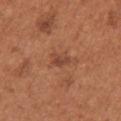Impression: This lesion was catalogued during total-body skin photography and was not selected for biopsy. Background: On the right upper arm. The recorded lesion diameter is about 2.5 mm. Automated image analysis of the tile measured a shape eccentricity near 0.8. The software also gave internal color variation of about 1.5 on a 0–10 scale and a peripheral color-asymmetry measure near 0.5. The software also gave an automated nevus-likeness rating near 5 out of 100 and a lesion-detection confidence of about 100/100. Imaged with white-light lighting. A female subject approximately 65 years of age. A 15 mm crop from a total-body photograph taken for skin-cancer surveillance.